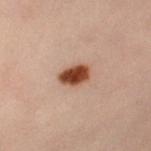A lesion tile, about 15 mm wide, cut from a 3D total-body photograph.
The lesion is on the right leg.
The tile uses cross-polarized illumination.
The subject is a female roughly 60 years of age.
The lesion's longest dimension is about 3.5 mm.